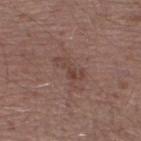| feature | finding |
|---|---|
| biopsy status | imaged on a skin check; not biopsied |
| subject | male, in their mid-40s |
| acquisition | ~15 mm tile from a whole-body skin photo |
| body site | the left lower leg |